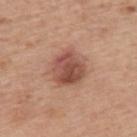Part of a total-body skin-imaging series; this lesion was reviewed on a skin check and was not flagged for biopsy. Located on the upper back. A close-up tile cropped from a whole-body skin photograph, about 15 mm across. The lesion's longest dimension is about 4.5 mm. This is a white-light tile. A male patient roughly 75 years of age.